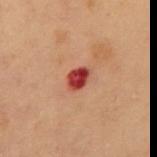{"biopsy_status": "not biopsied; imaged during a skin examination", "site": "abdomen", "patient": {"sex": "male", "age_approx": 55}, "lesion_size": {"long_diameter_mm_approx": 2.5}, "lighting": "cross-polarized", "image": {"source": "total-body photography crop", "field_of_view_mm": 15}, "automated_metrics": {"area_mm2_approx": 4.5, "eccentricity": 0.7, "shape_asymmetry": 0.2, "border_irregularity_0_10": 1.5, "color_variation_0_10": 4.0, "peripheral_color_asymmetry": 1.5, "nevus_likeness_0_100": 0}}